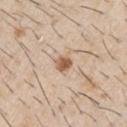workup = no biopsy performed (imaged during a skin exam) | image = ~15 mm tile from a whole-body skin photo | patient = male, roughly 60 years of age | anatomic site = the arm.